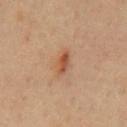Q: Was a biopsy performed?
A: total-body-photography surveillance lesion; no biopsy
Q: What kind of image is this?
A: 15 mm crop, total-body photography
Q: Who is the patient?
A: male, in their mid- to late 50s
Q: Lesion location?
A: the chest